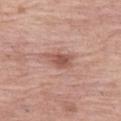Case summary:
– notes: no biopsy performed (imaged during a skin exam)
– automated lesion analysis: an eccentricity of roughly 0.8 and a shape-asymmetry score of about 0.35 (0 = symmetric); a color-variation rating of about 2.5/10 and peripheral color asymmetry of about 1
– image: ~15 mm crop, total-body skin-cancer survey
– tile lighting: white-light illumination
– diameter: ~3.5 mm (longest diameter)
– site: the left thigh
– patient: female, aged 63 to 67